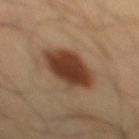Q: Is there a histopathology result?
A: imaged on a skin check; not biopsied
Q: Lesion location?
A: the mid back
Q: What are the patient's age and sex?
A: male, in their mid-50s
Q: How was this image acquired?
A: ~15 mm tile from a whole-body skin photo